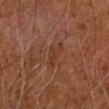follow-up=no biopsy performed (imaged during a skin exam) | site=the left arm | image source=total-body-photography crop, ~15 mm field of view | patient=male, aged around 60 | illumination=cross-polarized | lesion diameter=≈3.5 mm.